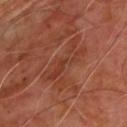biopsy status: no biopsy performed (imaged during a skin exam); patient: male, aged 58 to 62; site: the upper back; image source: total-body-photography crop, ~15 mm field of view.